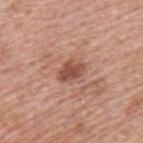diameter: ≈3 mm | imaging modality: ~15 mm crop, total-body skin-cancer survey | tile lighting: white-light | location: the upper back | patient: male, roughly 75 years of age.